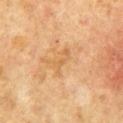Captured during whole-body skin photography for melanoma surveillance; the lesion was not biopsied. A male subject, aged 63–67. Located on the chest. A 15 mm close-up tile from a total-body photography series done for melanoma screening. Captured under cross-polarized illumination.